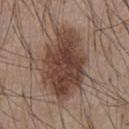Recorded during total-body skin imaging; not selected for excision or biopsy.
From the front of the torso.
A male subject, aged 53 to 57.
The tile uses white-light illumination.
The recorded lesion diameter is about 8.5 mm.
Cropped from a total-body skin-imaging series; the visible field is about 15 mm.